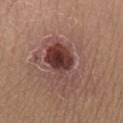Assessment: This lesion was catalogued during total-body skin photography and was not selected for biopsy. Background: The lesion is located on the left lower leg. A female patient in their mid- to late 30s. A lesion tile, about 15 mm wide, cut from a 3D total-body photograph.